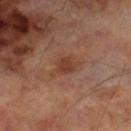This image is a 15 mm lesion crop taken from a total-body photograph. The lesion's longest dimension is about 3.5 mm. The lesion is located on the right lower leg. Captured under cross-polarized illumination. A male subject, in their 70s. An algorithmic analysis of the crop reported a lesion color around L≈39 a*≈22 b*≈29 in CIELAB and a normalized lesion–skin contrast near 6.5. The analysis additionally found a detector confidence of about 100 out of 100 that the crop contains a lesion.